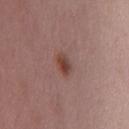Imaged during a routine full-body skin examination; the lesion was not biopsied and no histopathology is available. A female patient, in their 50s. Located on the chest. The tile uses white-light illumination. About 2.5 mm across. The total-body-photography lesion software estimated an area of roughly 3.5 mm² and an eccentricity of roughly 0.85. It also reported peripheral color asymmetry of about 1.5. The software also gave a detector confidence of about 100 out of 100 that the crop contains a lesion. A 15 mm close-up tile from a total-body photography series done for melanoma screening.